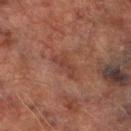The lesion was photographed on a routine skin check and not biopsied; there is no pathology result.
A male subject approximately 75 years of age.
Located on the right lower leg.
A close-up tile cropped from a whole-body skin photograph, about 15 mm across.
Imaged with cross-polarized lighting.